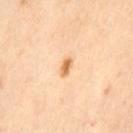Q: Is there a histopathology result?
A: no biopsy performed (imaged during a skin exam)
Q: What are the patient's age and sex?
A: female, aged 53–57
Q: How was the tile lit?
A: cross-polarized
Q: Lesion location?
A: the left thigh
Q: What is the lesion's diameter?
A: ≈2 mm
Q: What kind of image is this?
A: ~15 mm crop, total-body skin-cancer survey